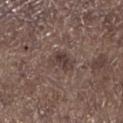The lesion was photographed on a routine skin check and not biopsied; there is no pathology result. The patient is a male aged approximately 70. From the left lower leg. A 15 mm crop from a total-body photograph taken for skin-cancer surveillance.